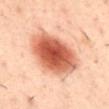<case>
<biopsy_status>not biopsied; imaged during a skin examination</biopsy_status>
<lighting>cross-polarized</lighting>
<image>
  <source>total-body photography crop</source>
  <field_of_view_mm>15</field_of_view_mm>
</image>
<automated_metrics>
  <area_mm2_approx>27.0</area_mm2_approx>
  <eccentricity>0.65</eccentricity>
  <shape_asymmetry>0.15</shape_asymmetry>
  <border_irregularity_0_10>1.5</border_irregularity_0_10>
  <color_variation_0_10>7.0</color_variation_0_10>
  <peripheral_color_asymmetry>1.5</peripheral_color_asymmetry>
  <nevus_likeness_0_100>100</nevus_likeness_0_100>
</automated_metrics>
<site>back</site>
<patient>
  <sex>male</sex>
  <age_approx>40</age_approx>
</patient>
<lesion_size>
  <long_diameter_mm_approx>6.5</long_diameter_mm_approx>
</lesion_size>
</case>